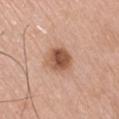Q: Was a biopsy performed?
A: catalogued during a skin exam; not biopsied
Q: What is the imaging modality?
A: total-body-photography crop, ~15 mm field of view
Q: Who is the patient?
A: male, in their mid-70s
Q: How was the tile lit?
A: white-light illumination
Q: How large is the lesion?
A: ~3.5 mm (longest diameter)
Q: What is the anatomic site?
A: the arm